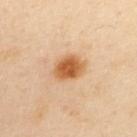{
  "biopsy_status": "not biopsied; imaged during a skin examination",
  "lesion_size": {
    "long_diameter_mm_approx": 4.0
  },
  "patient": {
    "sex": "female",
    "age_approx": 40
  },
  "automated_metrics": {
    "area_mm2_approx": 9.0,
    "eccentricity": 0.65,
    "cielab_L": 61,
    "cielab_a": 24,
    "cielab_b": 42,
    "vs_skin_darker_L": 14.0,
    "vs_skin_contrast_norm": 10.0,
    "border_irregularity_0_10": 2.0,
    "color_variation_0_10": 4.0,
    "peripheral_color_asymmetry": 1.0
  },
  "lighting": "cross-polarized",
  "site": "back",
  "image": {
    "source": "total-body photography crop",
    "field_of_view_mm": 15
  }
}